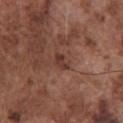Clinical impression:
No biopsy was performed on this lesion — it was imaged during a full skin examination and was not determined to be concerning.
Context:
Automated image analysis of the tile measured an area of roughly 3 mm², an eccentricity of roughly 0.8, and a symmetry-axis asymmetry near 0.25. The software also gave a border-irregularity rating of about 3/10 and peripheral color asymmetry of about 0.5. It also reported an automated nevus-likeness rating near 0 out of 100 and lesion-presence confidence of about 100/100. Longest diameter approximately 2.5 mm. Imaged with white-light lighting. On the chest. A lesion tile, about 15 mm wide, cut from a 3D total-body photograph. The patient is a male approximately 75 years of age.